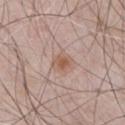Q: Was this lesion biopsied?
A: total-body-photography surveillance lesion; no biopsy
Q: What are the patient's age and sex?
A: male, in their mid-60s
Q: How was the tile lit?
A: white-light
Q: What kind of image is this?
A: ~15 mm tile from a whole-body skin photo
Q: What is the anatomic site?
A: the abdomen
Q: Lesion size?
A: about 2.5 mm